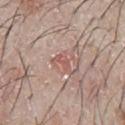Q: Is there a histopathology result?
A: total-body-photography surveillance lesion; no biopsy
Q: What is the imaging modality?
A: 15 mm crop, total-body photography
Q: Who is the patient?
A: male, about 65 years old
Q: Illumination type?
A: white-light illumination
Q: Automated lesion metrics?
A: a nevus-likeness score of about 0/100 and a lesion-detection confidence of about 95/100
Q: Lesion location?
A: the chest
Q: What is the lesion's diameter?
A: ≈2.5 mm